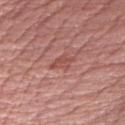The lesion was tiled from a total-body skin photograph and was not biopsied.
Cropped from a total-body skin-imaging series; the visible field is about 15 mm.
The lesion's longest dimension is about 3 mm.
A female patient approximately 70 years of age.
On the right upper arm.